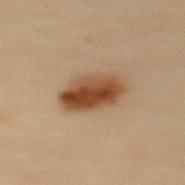This lesion was catalogued during total-body skin photography and was not selected for biopsy.
Measured at roughly 5 mm in maximum diameter.
The total-body-photography lesion software estimated a footprint of about 14 mm², an eccentricity of roughly 0.75, and two-axis asymmetry of about 0.15. The analysis additionally found a mean CIELAB color near L≈41 a*≈18 b*≈30, roughly 13 lightness units darker than nearby skin, and a lesion-to-skin contrast of about 11 (normalized; higher = more distinct).
On the upper back.
A female subject about 60 years old.
This image is a 15 mm lesion crop taken from a total-body photograph.
This is a cross-polarized tile.